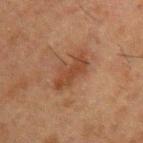Q: Is there a histopathology result?
A: catalogued during a skin exam; not biopsied
Q: What is the imaging modality?
A: ~15 mm tile from a whole-body skin photo
Q: Lesion location?
A: the left upper arm
Q: What lighting was used for the tile?
A: cross-polarized illumination
Q: Automated lesion metrics?
A: a lesion–skin lightness drop of about 7 and a lesion-to-skin contrast of about 7 (normalized; higher = more distinct); a classifier nevus-likeness of about 5/100 and a lesion-detection confidence of about 100/100
Q: Patient demographics?
A: male, aged around 50